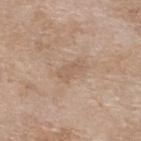Q: Was this lesion biopsied?
A: catalogued during a skin exam; not biopsied
Q: What did automated image analysis measure?
A: an area of roughly 5 mm² and a shape-asymmetry score of about 0.25 (0 = symmetric); a lesion color around L≈59 a*≈16 b*≈29 in CIELAB and a lesion–skin lightness drop of about 6; a border-irregularity rating of about 3/10 and radial color variation of about 0.5; a classifier nevus-likeness of about 0/100 and lesion-presence confidence of about 100/100
Q: Where on the body is the lesion?
A: the back
Q: What kind of image is this?
A: total-body-photography crop, ~15 mm field of view
Q: How large is the lesion?
A: ≈3.5 mm
Q: What are the patient's age and sex?
A: female, roughly 75 years of age